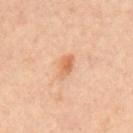{
  "biopsy_status": "not biopsied; imaged during a skin examination",
  "site": "mid back",
  "patient": {
    "sex": "female",
    "age_approx": 45
  },
  "lesion_size": {
    "long_diameter_mm_approx": 3.0
  },
  "lighting": "cross-polarized",
  "image": {
    "source": "total-body photography crop",
    "field_of_view_mm": 15
  }
}